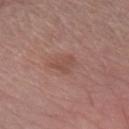Imaged during a routine full-body skin examination; the lesion was not biopsied and no histopathology is available. A male patient aged approximately 55. This image is a 15 mm lesion crop taken from a total-body photograph. On the arm.